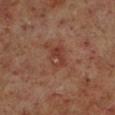The lesion was photographed on a routine skin check and not biopsied; there is no pathology result.
The recorded lesion diameter is about 2.5 mm.
Captured under cross-polarized illumination.
A male patient aged around 60.
The lesion is located on the left lower leg.
A 15 mm close-up extracted from a 3D total-body photography capture.